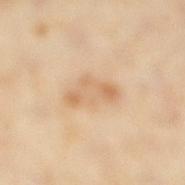- notes — imaged on a skin check; not biopsied
- body site — the right lower leg
- automated metrics — an area of roughly 9.5 mm², a shape eccentricity near 0.9, and a shape-asymmetry score of about 0.35 (0 = symmetric)
- lesion diameter — ~5 mm (longest diameter)
- imaging modality — total-body-photography crop, ~15 mm field of view
- tile lighting — cross-polarized illumination
- subject — female, aged 33 to 37